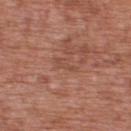biopsy status = catalogued during a skin exam; not biopsied.